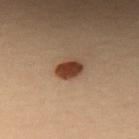Case summary:
- notes: imaged on a skin check; not biopsied
- image: 15 mm crop, total-body photography
- diameter: ≈3 mm
- location: the upper back
- subject: female, aged approximately 30
- illumination: cross-polarized illumination